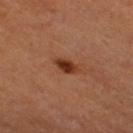Assessment: Imaged during a routine full-body skin examination; the lesion was not biopsied and no histopathology is available. Acquisition and patient details: The lesion-visualizer software estimated an outline eccentricity of about 0.8 (0 = round, 1 = elongated). And it measured a border-irregularity index near 2.5/10, internal color variation of about 3.5 on a 0–10 scale, and peripheral color asymmetry of about 1. The subject is a female approximately 50 years of age. A region of skin cropped from a whole-body photographic capture, roughly 15 mm wide.